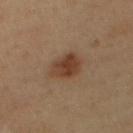Imaged during a routine full-body skin examination; the lesion was not biopsied and no histopathology is available. A lesion tile, about 15 mm wide, cut from a 3D total-body photograph. A male patient, about 50 years old. Measured at roughly 3.5 mm in maximum diameter. The lesion is on the abdomen. The lesion-visualizer software estimated a mean CIELAB color near L≈39 a*≈19 b*≈30, about 10 CIELAB-L* units darker than the surrounding skin, and a normalized lesion–skin contrast near 9. The analysis additionally found a border-irregularity index near 2/10 and a peripheral color-asymmetry measure near 1.5.